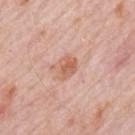{"biopsy_status": "not biopsied; imaged during a skin examination", "image": {"source": "total-body photography crop", "field_of_view_mm": 15}, "lighting": "white-light", "site": "back", "lesion_size": {"long_diameter_mm_approx": 3.0}, "patient": {"sex": "male", "age_approx": 80}, "automated_metrics": {"eccentricity": 0.75, "peripheral_color_asymmetry": 1.5, "nevus_likeness_0_100": 15}}